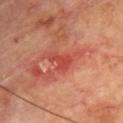| feature | finding |
|---|---|
| biopsy status | catalogued during a skin exam; not biopsied |
| lesion diameter | ~3 mm (longest diameter) |
| subject | male, in their 70s |
| tile lighting | cross-polarized |
| acquisition | total-body-photography crop, ~15 mm field of view |
| body site | the chest |